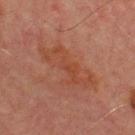Assessment: This lesion was catalogued during total-body skin photography and was not selected for biopsy. Acquisition and patient details: A region of skin cropped from a whole-body photographic capture, roughly 15 mm wide. An algorithmic analysis of the crop reported a footprint of about 14 mm², an eccentricity of roughly 0.9, and a shape-asymmetry score of about 0.55 (0 = symmetric). The software also gave a border-irregularity rating of about 8.5/10, internal color variation of about 3 on a 0–10 scale, and peripheral color asymmetry of about 1. It also reported a lesion-detection confidence of about 100/100. The subject is a male aged around 70. The lesion is on the chest. Captured under cross-polarized illumination. About 7 mm across.